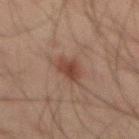  biopsy_status: not biopsied; imaged during a skin examination
  site: abdomen
  lighting: cross-polarized
  image:
    source: total-body photography crop
    field_of_view_mm: 15
  patient:
    sex: male
    age_approx: 45